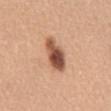Q: What kind of image is this?
A: 15 mm crop, total-body photography
Q: How large is the lesion?
A: ~4.5 mm (longest diameter)
Q: What are the patient's age and sex?
A: female, in their mid-60s
Q: What lighting was used for the tile?
A: white-light
Q: What is the anatomic site?
A: the mid back
Q: What did automated image analysis measure?
A: an average lesion color of about L≈52 a*≈23 b*≈32 (CIELAB), roughly 18 lightness units darker than nearby skin, and a normalized border contrast of about 11.5